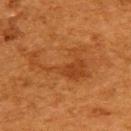The lesion's longest dimension is about 7.5 mm.
Located on the back.
Cropped from a whole-body photographic skin survey; the tile spans about 15 mm.
The lesion-visualizer software estimated a nevus-likeness score of about 0/100 and lesion-presence confidence of about 100/100.
A female patient aged around 50.
The tile uses cross-polarized illumination.